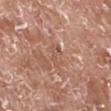Part of a total-body skin-imaging series; this lesion was reviewed on a skin check and was not flagged for biopsy. A close-up tile cropped from a whole-body skin photograph, about 15 mm across. Measured at roughly 2.5 mm in maximum diameter. The lesion is located on the right lower leg. A male subject roughly 75 years of age.